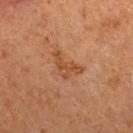Automated tile analysis of the lesion measured a mean CIELAB color near L≈41 a*≈21 b*≈33, roughly 7 lightness units darker than nearby skin, and a lesion-to-skin contrast of about 7 (normalized; higher = more distinct). It also reported a classifier nevus-likeness of about 0/100 and a lesion-detection confidence of about 100/100. A male patient approximately 65 years of age. About 4 mm across. On the mid back. Imaged with cross-polarized lighting. A region of skin cropped from a whole-body photographic capture, roughly 15 mm wide.